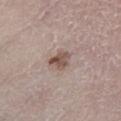Q: Is there a histopathology result?
A: catalogued during a skin exam; not biopsied
Q: What is the anatomic site?
A: the left lower leg
Q: How was the tile lit?
A: white-light illumination
Q: How was this image acquired?
A: total-body-photography crop, ~15 mm field of view
Q: What is the lesion's diameter?
A: about 3 mm
Q: Who is the patient?
A: male, about 75 years old